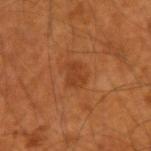{"patient": {"sex": "male", "age_approx": 55}, "image": {"source": "total-body photography crop", "field_of_view_mm": 15}, "site": "right forearm"}